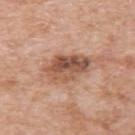Recorded during total-body skin imaging; not selected for excision or biopsy. A male subject, about 60 years old. The lesion is located on the left upper arm. This image is a 15 mm lesion crop taken from a total-body photograph. The lesion's longest dimension is about 5 mm.